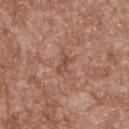  biopsy_status: not biopsied; imaged during a skin examination
  patient:
    sex: male
    age_approx: 45
  site: upper back
  lighting: white-light
  image:
    source: total-body photography crop
    field_of_view_mm: 15